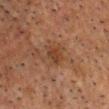Notes:
– notes: no biopsy performed (imaged during a skin exam)
– imaging modality: total-body-photography crop, ~15 mm field of view
– anatomic site: the head or neck
– patient: male, aged 58 to 62
– automated metrics: about 6 CIELAB-L* units darker than the surrounding skin and a normalized lesion–skin contrast near 6.5; an automated nevus-likeness rating near 0 out of 100 and a detector confidence of about 100 out of 100 that the crop contains a lesion
– diameter: about 3 mm
– tile lighting: cross-polarized